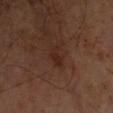• workup — total-body-photography surveillance lesion; no biopsy
• subject — male, aged 63–67
• imaging modality — 15 mm crop, total-body photography
• diameter — ~3 mm (longest diameter)
• image-analysis metrics — a lesion area of about 3.5 mm² and two-axis asymmetry of about 0.35; a mean CIELAB color near L≈24 a*≈19 b*≈22, about 5 CIELAB-L* units darker than the surrounding skin, and a normalized border contrast of about 6; a color-variation rating of about 0.5/10 and peripheral color asymmetry of about 0
• location — the left forearm
• illumination — cross-polarized illumination